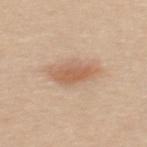Q: Was this lesion biopsied?
A: imaged on a skin check; not biopsied
Q: How large is the lesion?
A: ~4.5 mm (longest diameter)
Q: Who is the patient?
A: female, about 40 years old
Q: What is the anatomic site?
A: the mid back
Q: How was this image acquired?
A: 15 mm crop, total-body photography